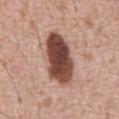biopsy status = imaged on a skin check; not biopsied
patient = male, aged approximately 60
size = ~7 mm (longest diameter)
image source = 15 mm crop, total-body photography
body site = the abdomen
TBP lesion metrics = a mean CIELAB color near L≈46 a*≈22 b*≈25, about 21 CIELAB-L* units darker than the surrounding skin, and a normalized lesion–skin contrast near 14
illumination = white-light illumination